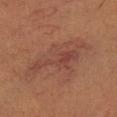{"site": "left leg", "lesion_size": {"long_diameter_mm_approx": 8.5}, "lighting": "cross-polarized", "patient": {"sex": "female", "age_approx": 45}, "image": {"source": "total-body photography crop", "field_of_view_mm": 15}}